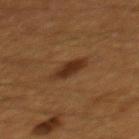| feature | finding |
|---|---|
| notes | total-body-photography surveillance lesion; no biopsy |
| tile lighting | cross-polarized |
| lesion diameter | ~4 mm (longest diameter) |
| subject | male, aged 58 to 62 |
| site | the mid back |
| acquisition | ~15 mm tile from a whole-body skin photo |